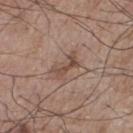| field | value |
|---|---|
| biopsy status | catalogued during a skin exam; not biopsied |
| automated metrics | a footprint of about 4.5 mm², an eccentricity of roughly 0.9, and a symmetry-axis asymmetry near 0.55; a mean CIELAB color near L≈48 a*≈17 b*≈26 and about 10 CIELAB-L* units darker than the surrounding skin; a color-variation rating of about 0/10 and peripheral color asymmetry of about 0; an automated nevus-likeness rating near 5 out of 100 and lesion-presence confidence of about 100/100 |
| image | total-body-photography crop, ~15 mm field of view |
| patient | male, roughly 75 years of age |
| anatomic site | the chest |